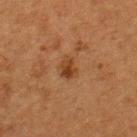  biopsy_status: not biopsied; imaged during a skin examination
  lesion_size:
    long_diameter_mm_approx: 3.0
  lighting: cross-polarized
  image:
    source: total-body photography crop
    field_of_view_mm: 15
  automated_metrics:
    area_mm2_approx: 4.5
    shape_asymmetry: 0.3
    vs_skin_darker_L: 8.0
    vs_skin_contrast_norm: 7.5
    border_irregularity_0_10: 3.0
    color_variation_0_10: 3.5
    peripheral_color_asymmetry: 1.0
  patient:
    sex: male
    age_approx: 50
  site: upper back